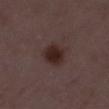| key | value |
|---|---|
| notes | no biopsy performed (imaged during a skin exam) |
| location | the left thigh |
| diameter | about 3 mm |
| subject | female, aged around 35 |
| image | ~15 mm crop, total-body skin-cancer survey |
| tile lighting | white-light illumination |